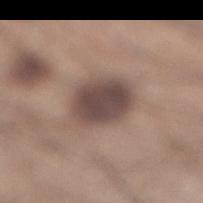Recorded during total-body skin imaging; not selected for excision or biopsy. Cropped from a whole-body photographic skin survey; the tile spans about 15 mm. The patient is a male approximately 35 years of age. On the right lower leg.